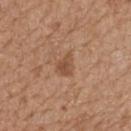| field | value |
|---|---|
| notes | catalogued during a skin exam; not biopsied |